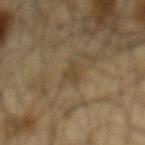The lesion was tiled from a total-body skin photograph and was not biopsied.
This image is a 15 mm lesion crop taken from a total-body photograph.
The tile uses cross-polarized illumination.
On the mid back.
A male patient aged around 65.